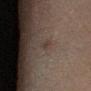  biopsy_status: not biopsied; imaged during a skin examination
  site: left lower leg
  lighting: cross-polarized
  automated_metrics:
    area_mm2_approx: 1.5
    eccentricity: 0.8
    shape_asymmetry: 0.5
    border_irregularity_0_10: 4.5
    color_variation_0_10: 0.0
    peripheral_color_asymmetry: 0.0
    nevus_likeness_0_100: 0
    lesion_detection_confidence_0_100: 100
  lesion_size:
    long_diameter_mm_approx: 1.5
  image:
    source: total-body photography crop
    field_of_view_mm: 15
  patient:
    sex: female
    age_approx: 55